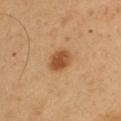Q: Is there a histopathology result?
A: total-body-photography surveillance lesion; no biopsy
Q: Automated lesion metrics?
A: a lesion color around L≈49 a*≈22 b*≈37 in CIELAB, a lesion–skin lightness drop of about 12, and a lesion-to-skin contrast of about 8.5 (normalized; higher = more distinct); internal color variation of about 3 on a 0–10 scale and a peripheral color-asymmetry measure near 1; an automated nevus-likeness rating near 100 out of 100 and a detector confidence of about 100 out of 100 that the crop contains a lesion
Q: What kind of image is this?
A: 15 mm crop, total-body photography
Q: What are the patient's age and sex?
A: male, aged around 55
Q: Illumination type?
A: cross-polarized illumination
Q: Lesion location?
A: the arm